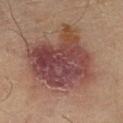follow-up: imaged on a skin check; not biopsied
image: ~15 mm tile from a whole-body skin photo
subject: female, approximately 70 years of age
tile lighting: cross-polarized illumination
anatomic site: the leg
lesion diameter: ≈9.5 mm
automated lesion analysis: a lesion color around L≈46 a*≈22 b*≈24 in CIELAB, about 12 CIELAB-L* units darker than the surrounding skin, and a lesion-to-skin contrast of about 9.5 (normalized; higher = more distinct); a classifier nevus-likeness of about 40/100 and a detector confidence of about 100 out of 100 that the crop contains a lesion